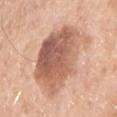biopsy status — no biopsy performed (imaged during a skin exam); anatomic site — the chest; subject — male, aged 68–72; illumination — white-light; size — ~8.5 mm (longest diameter); acquisition — ~15 mm crop, total-body skin-cancer survey; automated lesion analysis — an outline eccentricity of about 0.65 (0 = round, 1 = elongated) and a symmetry-axis asymmetry near 0.2.